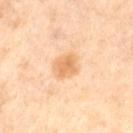Clinical impression:
No biopsy was performed on this lesion — it was imaged during a full skin examination and was not determined to be concerning.
Context:
On the right thigh. A 15 mm close-up extracted from a 3D total-body photography capture. A female subject, aged approximately 50. Imaged with cross-polarized lighting. Longest diameter approximately 2.5 mm.